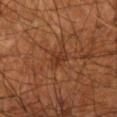Part of a total-body skin-imaging series; this lesion was reviewed on a skin check and was not flagged for biopsy.
Longest diameter approximately 3 mm.
Cropped from a total-body skin-imaging series; the visible field is about 15 mm.
Captured under cross-polarized illumination.
A male subject, in their mid-50s.
On the right forearm.
Automated image analysis of the tile measured about 6 CIELAB-L* units darker than the surrounding skin and a normalized lesion–skin contrast near 6. The analysis additionally found a border-irregularity index near 4.5/10 and internal color variation of about 2 on a 0–10 scale.